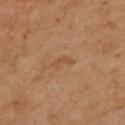{"biopsy_status": "not biopsied; imaged during a skin examination", "patient": {"sex": "female", "age_approx": 70}, "lesion_size": {"long_diameter_mm_approx": 2.5}, "site": "back", "image": {"source": "total-body photography crop", "field_of_view_mm": 15}, "automated_metrics": {"border_irregularity_0_10": 5.5, "color_variation_0_10": 0.0, "nevus_likeness_0_100": 0, "lesion_detection_confidence_0_100": 100}}